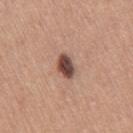biopsy_status: not biopsied; imaged during a skin examination
patient:
  sex: female
  age_approx: 55
lesion_size:
  long_diameter_mm_approx: 3.5
site: right thigh
automated_metrics:
  nevus_likeness_0_100: 95
  lesion_detection_confidence_0_100: 100
image:
  source: total-body photography crop
  field_of_view_mm: 15
lighting: white-light